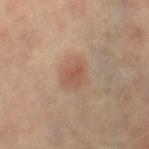<lesion>
<biopsy_status>not biopsied; imaged during a skin examination</biopsy_status>
<automated_metrics>
  <area_mm2_approx>9.0</area_mm2_approx>
  <eccentricity>0.45</eccentricity>
  <vs_skin_darker_L>7.0</vs_skin_darker_L>
  <vs_skin_contrast_norm>5.5</vs_skin_contrast_norm>
</automated_metrics>
<patient>
  <sex>female</sex>
  <age_approx>65</age_approx>
</patient>
<lesion_size>
  <long_diameter_mm_approx>3.5</long_diameter_mm_approx>
</lesion_size>
<image>
  <source>total-body photography crop</source>
  <field_of_view_mm>15</field_of_view_mm>
</image>
<lighting>cross-polarized</lighting>
<site>leg</site>
</lesion>